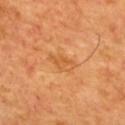Imaged during a routine full-body skin examination; the lesion was not biopsied and no histopathology is available.
This image is a 15 mm lesion crop taken from a total-body photograph.
On the upper back.
A male patient, aged around 65.
Captured under cross-polarized illumination.